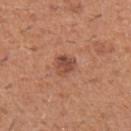follow-up — catalogued during a skin exam; not biopsied
subject — male, approximately 40 years of age
automated lesion analysis — an average lesion color of about L≈48 a*≈25 b*≈29 (CIELAB) and a normalized border contrast of about 7.5; a classifier nevus-likeness of about 40/100
lighting — white-light
image — total-body-photography crop, ~15 mm field of view
site — the left forearm